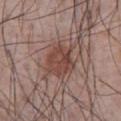workup=total-body-photography surveillance lesion; no biopsy
illumination=white-light
imaging modality=15 mm crop, total-body photography
subject=male, approximately 65 years of age
TBP lesion metrics=an area of roughly 12 mm² and a symmetry-axis asymmetry near 0.25; a normalized lesion–skin contrast near 7.5; border irregularity of about 2.5 on a 0–10 scale and a peripheral color-asymmetry measure near 1.5; an automated nevus-likeness rating near 85 out of 100 and lesion-presence confidence of about 100/100
location=the front of the torso